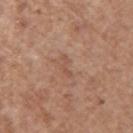Captured during whole-body skin photography for melanoma surveillance; the lesion was not biopsied.
A 15 mm crop from a total-body photograph taken for skin-cancer surveillance.
A female patient, aged approximately 65.
Measured at roughly 3 mm in maximum diameter.
Located on the arm.
The total-body-photography lesion software estimated a mean CIELAB color near L≈52 a*≈21 b*≈29 and a lesion-to-skin contrast of about 5 (normalized; higher = more distinct). And it measured a border-irregularity index near 7.5/10 and a within-lesion color-variation index near 0/10.
The tile uses white-light illumination.